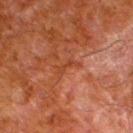{"biopsy_status": "not biopsied; imaged during a skin examination", "image": {"source": "total-body photography crop", "field_of_view_mm": 15}, "patient": {"sex": "male", "age_approx": 80}, "site": "leg", "lesion_size": {"long_diameter_mm_approx": 3.0}}